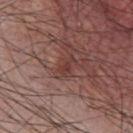The lesion was photographed on a routine skin check and not biopsied; there is no pathology result.
Longest diameter approximately 3 mm.
The patient is a male about 60 years old.
Imaged with white-light lighting.
On the chest.
Cropped from a total-body skin-imaging series; the visible field is about 15 mm.
Automated image analysis of the tile measured an area of roughly 3.5 mm² and a shape eccentricity near 0.85. The analysis additionally found a mean CIELAB color near L≈38 a*≈22 b*≈23, about 7 CIELAB-L* units darker than the surrounding skin, and a lesion-to-skin contrast of about 6 (normalized; higher = more distinct). The analysis additionally found a classifier nevus-likeness of about 0/100 and lesion-presence confidence of about 95/100.